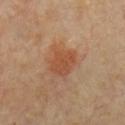The lesion was tiled from a total-body skin photograph and was not biopsied. The lesion is located on the chest. A female patient approximately 60 years of age. The lesion-visualizer software estimated an area of roughly 9.5 mm², an outline eccentricity of about 0.5 (0 = round, 1 = elongated), and two-axis asymmetry of about 0.25. A roughly 15 mm field-of-view crop from a total-body skin photograph. The tile uses cross-polarized illumination.